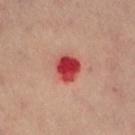Q: Was this lesion biopsied?
A: imaged on a skin check; not biopsied
Q: How large is the lesion?
A: ≈3.5 mm
Q: Where on the body is the lesion?
A: the abdomen
Q: How was this image acquired?
A: 15 mm crop, total-body photography
Q: What did automated image analysis measure?
A: a mean CIELAB color near L≈47 a*≈42 b*≈30 and about 17 CIELAB-L* units darker than the surrounding skin; a classifier nevus-likeness of about 0/100
Q: Who is the patient?
A: female, aged approximately 40